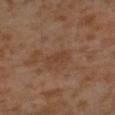follow-up = catalogued during a skin exam; not biopsied | anatomic site = the left lower leg | acquisition = ~15 mm crop, total-body skin-cancer survey | patient = male, aged approximately 30.